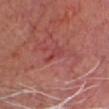Impression: The lesion was photographed on a routine skin check and not biopsied; there is no pathology result. Context: This is a cross-polarized tile. Automated tile analysis of the lesion measured a lesion area of about 3 mm², a shape eccentricity near 0.85, and a symmetry-axis asymmetry near 0.5. And it measured a mean CIELAB color near L≈41 a*≈31 b*≈22, about 6 CIELAB-L* units darker than the surrounding skin, and a lesion-to-skin contrast of about 5 (normalized; higher = more distinct). The analysis additionally found a border-irregularity index near 5/10, a within-lesion color-variation index near 0/10, and radial color variation of about 0. The software also gave a classifier nevus-likeness of about 0/100 and lesion-presence confidence of about 80/100. A lesion tile, about 15 mm wide, cut from a 3D total-body photograph. The subject is a male aged 58–62. The lesion is on the head or neck.